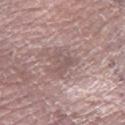– biopsy status: no biopsy performed (imaged during a skin exam)